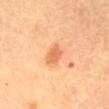Impression:
No biopsy was performed on this lesion — it was imaged during a full skin examination and was not determined to be concerning.
Background:
A 15 mm close-up tile from a total-body photography series done for melanoma screening. About 3 mm across. The patient is a female in their 60s. Imaged with cross-polarized lighting. The lesion is located on the abdomen. The total-body-photography lesion software estimated an area of roughly 4 mm², an eccentricity of roughly 0.75, and a symmetry-axis asymmetry near 0.25. The analysis additionally found a border-irregularity index near 2/10, a color-variation rating of about 1.5/10, and peripheral color asymmetry of about 0.5. The software also gave a lesion-detection confidence of about 100/100.